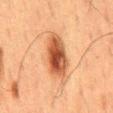Q: Was a biopsy performed?
A: catalogued during a skin exam; not biopsied